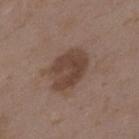Impression:
Part of a total-body skin-imaging series; this lesion was reviewed on a skin check and was not flagged for biopsy.
Acquisition and patient details:
Located on the upper back. A female subject, in their mid- to late 30s. A region of skin cropped from a whole-body photographic capture, roughly 15 mm wide.